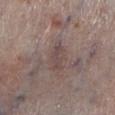• image · total-body-photography crop, ~15 mm field of view
• location · the left lower leg
• tile lighting · white-light illumination
• subject · male, aged around 80
• image-analysis metrics · a lesion area of about 6 mm², an outline eccentricity of about 0.85 (0 = round, 1 = elongated), and a symmetry-axis asymmetry near 0.25; an average lesion color of about L≈46 a*≈15 b*≈17 (CIELAB) and about 7 CIELAB-L* units darker than the surrounding skin
• size · ≈3.5 mm